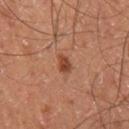Assessment:
Captured during whole-body skin photography for melanoma surveillance; the lesion was not biopsied.